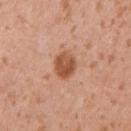workup = imaged on a skin check; not biopsied
illumination = white-light illumination
anatomic site = the left upper arm
imaging modality = ~15 mm tile from a whole-body skin photo
lesion diameter = ≈3.5 mm
subject = male, about 35 years old